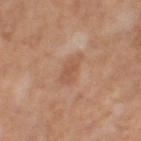* notes: no biopsy performed (imaged during a skin exam)
* patient: female, aged 48 to 52
* illumination: cross-polarized
* image: ~15 mm tile from a whole-body skin photo
* TBP lesion metrics: a within-lesion color-variation index near 2/10 and peripheral color asymmetry of about 0.5
* size: ≈3.5 mm
* anatomic site: the left thigh